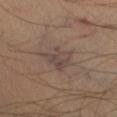Q: Is there a histopathology result?
A: catalogued during a skin exam; not biopsied
Q: What is the anatomic site?
A: the leg
Q: How was this image acquired?
A: ~15 mm crop, total-body skin-cancer survey
Q: Patient demographics?
A: male, approximately 45 years of age